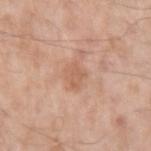Notes:
• follow-up: imaged on a skin check; not biopsied
• imaging modality: 15 mm crop, total-body photography
• illumination: white-light illumination
• patient: male, aged 53 to 57
• TBP lesion metrics: a footprint of about 4.5 mm², an eccentricity of roughly 0.65, and a symmetry-axis asymmetry near 0.3; a lesion color around L≈60 a*≈22 b*≈32 in CIELAB, a lesion–skin lightness drop of about 7, and a lesion-to-skin contrast of about 5.5 (normalized; higher = more distinct)
• body site: the arm